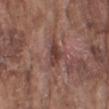workup: total-body-photography surveillance lesion; no biopsy | site: the arm | lighting: white-light illumination | subject: male, aged 73–77 | image: total-body-photography crop, ~15 mm field of view.